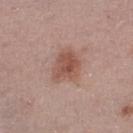Clinical impression:
Recorded during total-body skin imaging; not selected for excision or biopsy.
Acquisition and patient details:
About 4 mm across. A 15 mm close-up extracted from a 3D total-body photography capture. A male patient, roughly 75 years of age. On the left lower leg. Captured under white-light illumination. Automated image analysis of the tile measured a footprint of about 10 mm², a shape eccentricity near 0.6, and two-axis asymmetry of about 0.25. The analysis additionally found border irregularity of about 2.5 on a 0–10 scale, a color-variation rating of about 4/10, and a peripheral color-asymmetry measure near 1.5.